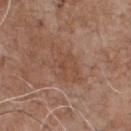{
  "biopsy_status": "not biopsied; imaged during a skin examination",
  "site": "chest",
  "lesion_size": {
    "long_diameter_mm_approx": 6.0
  },
  "automated_metrics": {
    "area_mm2_approx": 11.0,
    "eccentricity": 0.85
  },
  "lighting": "white-light",
  "patient": {
    "sex": "male",
    "age_approx": 70
  },
  "image": {
    "source": "total-body photography crop",
    "field_of_view_mm": 15
  }
}